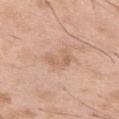The lesion was photographed on a routine skin check and not biopsied; there is no pathology result.